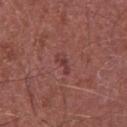Captured during whole-body skin photography for melanoma surveillance; the lesion was not biopsied.
Captured under white-light illumination.
This image is a 15 mm lesion crop taken from a total-body photograph.
Located on the front of the torso.
Measured at roughly 2.5 mm in maximum diameter.
The subject is a male aged around 65.